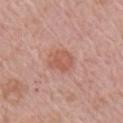<record>
<biopsy_status>not biopsied; imaged during a skin examination</biopsy_status>
<lesion_size>
  <long_diameter_mm_approx>3.0</long_diameter_mm_approx>
</lesion_size>
<site>right upper arm</site>
<image>
  <source>total-body photography crop</source>
  <field_of_view_mm>15</field_of_view_mm>
</image>
<patient>
  <sex>female</sex>
  <age_approx>65</age_approx>
</patient>
<automated_metrics>
  <area_mm2_approx>6.5</area_mm2_approx>
  <eccentricity>0.5</eccentricity>
  <cielab_L>57</cielab_L>
  <cielab_a>25</cielab_a>
  <cielab_b>29</cielab_b>
  <vs_skin_darker_L>8.0</vs_skin_darker_L>
  <vs_skin_contrast_norm>6.0</vs_skin_contrast_norm>
  <border_irregularity_0_10>1.5</border_irregularity_0_10>
  <color_variation_0_10>2.5</color_variation_0_10>
  <peripheral_color_asymmetry>1.0</peripheral_color_asymmetry>
</automated_metrics>
<lighting>white-light</lighting>
</record>